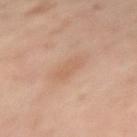The lesion was tiled from a total-body skin photograph and was not biopsied.
A 15 mm close-up extracted from a 3D total-body photography capture.
A female patient in their mid-50s.
Located on the left upper arm.